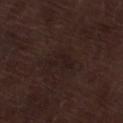workup: no biopsy performed (imaged during a skin exam) | illumination: white-light | image: ~15 mm tile from a whole-body skin photo | lesion diameter: about 3.5 mm | subject: male, aged around 70 | body site: the left lower leg | automated metrics: a lesion color around L≈17 a*≈12 b*≈14 in CIELAB, about 3 CIELAB-L* units darker than the surrounding skin, and a normalized border contrast of about 5.5; a classifier nevus-likeness of about 0/100 and a lesion-detection confidence of about 100/100.